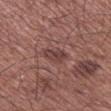Findings:
* automated lesion analysis · a lesion color around L≈41 a*≈21 b*≈20 in CIELAB; a border-irregularity index near 3/10, a color-variation rating of about 3/10, and a peripheral color-asymmetry measure near 1
* size · ~3.5 mm (longest diameter)
* body site · the right lower leg
* imaging modality · ~15 mm tile from a whole-body skin photo
* tile lighting · white-light illumination
* patient · male, in their mid- to late 60s